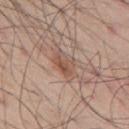Findings:
- follow-up — total-body-photography surveillance lesion; no biopsy
- subject — male, about 55 years old
- site — the mid back
- image-analysis metrics — two-axis asymmetry of about 0.25; border irregularity of about 3 on a 0–10 scale and a color-variation rating of about 4/10
- image source — total-body-photography crop, ~15 mm field of view